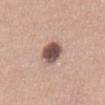Captured during whole-body skin photography for melanoma surveillance; the lesion was not biopsied. The recorded lesion diameter is about 3 mm. The lesion-visualizer software estimated a mean CIELAB color near L≈50 a*≈18 b*≈23, a lesion–skin lightness drop of about 19, and a normalized lesion–skin contrast near 12.5. The software also gave a border-irregularity rating of about 1.5/10, a color-variation rating of about 3.5/10, and peripheral color asymmetry of about 1. The subject is a female in their mid-40s. A 15 mm crop from a total-body photograph taken for skin-cancer surveillance. The lesion is on the abdomen.